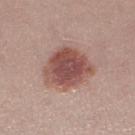workup — no biopsy performed (imaged during a skin exam); patient — female, in their mid-20s; body site — the left thigh; acquisition — total-body-photography crop, ~15 mm field of view.